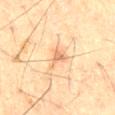{"biopsy_status": "not biopsied; imaged during a skin examination", "lesion_size": {"long_diameter_mm_approx": 4.5}, "lighting": "cross-polarized", "patient": {"sex": "male", "age_approx": 60}, "image": {"source": "total-body photography crop", "field_of_view_mm": 15}}